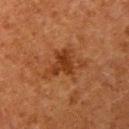Assessment: The lesion was tiled from a total-body skin photograph and was not biopsied. Context: From the left upper arm. This image is a 15 mm lesion crop taken from a total-body photograph. The patient is a female about 50 years old.